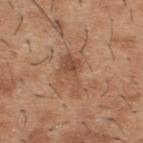<tbp_lesion>
<patient>
  <sex>male</sex>
  <age_approx>40</age_approx>
</patient>
<image>
  <source>total-body photography crop</source>
  <field_of_view_mm>15</field_of_view_mm>
</image>
<automated_metrics>
  <cielab_L>50</cielab_L>
  <cielab_a>21</cielab_a>
  <cielab_b>32</cielab_b>
  <vs_skin_contrast_norm>6.0</vs_skin_contrast_norm>
  <color_variation_0_10>4.0</color_variation_0_10>
  <peripheral_color_asymmetry>1.5</peripheral_color_asymmetry>
  <nevus_likeness_0_100>0</nevus_likeness_0_100>
  <lesion_detection_confidence_0_100>100</lesion_detection_confidence_0_100>
</automated_metrics>
<site>upper back</site>
</tbp_lesion>